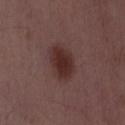A male subject, aged approximately 50. A 15 mm close-up tile from a total-body photography series done for melanoma screening.